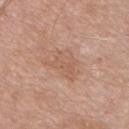Notes:
– biopsy status: imaged on a skin check; not biopsied
– TBP lesion metrics: a lesion area of about 8 mm², an outline eccentricity of about 0.55 (0 = round, 1 = elongated), and a shape-asymmetry score of about 0.5 (0 = symmetric); an average lesion color of about L≈58 a*≈20 b*≈30 (CIELAB), roughly 6 lightness units darker than nearby skin, and a lesion-to-skin contrast of about 4.5 (normalized; higher = more distinct); a classifier nevus-likeness of about 0/100
– image source: 15 mm crop, total-body photography
– site: the front of the torso
– patient: male, aged approximately 75
– lighting: white-light illumination